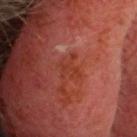Assessment:
Recorded during total-body skin imaging; not selected for excision or biopsy.